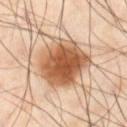  biopsy_status: not biopsied; imaged during a skin examination
  automated_metrics:
    cielab_L: 58
    cielab_a: 22
    cielab_b: 36
    vs_skin_darker_L: 17.0
    vs_skin_contrast_norm: 11.0
    border_irregularity_0_10: 2.0
    color_variation_0_10: 7.0
    peripheral_color_asymmetry: 2.0
  site: leg
  image:
    source: total-body photography crop
    field_of_view_mm: 15
  patient:
    sex: male
    age_approx: 55
  lighting: cross-polarized
  lesion_size:
    long_diameter_mm_approx: 7.0